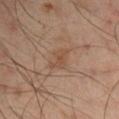  biopsy_status: not biopsied; imaged during a skin examination
  image:
    source: total-body photography crop
    field_of_view_mm: 15
  patient:
    sex: male
    age_approx: 50
  lighting: cross-polarized
  automated_metrics:
    border_irregularity_0_10: 3.5
    color_variation_0_10: 2.0
    peripheral_color_asymmetry: 0.5
  site: left arm